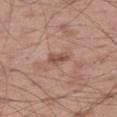Clinical impression: Imaged during a routine full-body skin examination; the lesion was not biopsied and no histopathology is available. Image and clinical context: Cropped from a whole-body photographic skin survey; the tile spans about 15 mm. Approximately 2.5 mm at its widest. The lesion-visualizer software estimated a lesion color around L≈51 a*≈21 b*≈27 in CIELAB and about 10 CIELAB-L* units darker than the surrounding skin. It also reported a border-irregularity index near 3.5/10, internal color variation of about 1.5 on a 0–10 scale, and radial color variation of about 0.5. The lesion is located on the leg. The patient is a male in their mid- to late 50s.